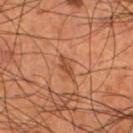image-analysis metrics: an automated nevus-likeness rating near 20 out of 100 and a lesion-detection confidence of about 95/100
patient: male, in their mid-50s
diameter: ≈2.5 mm
site: the left lower leg
image: ~15 mm crop, total-body skin-cancer survey
tile lighting: cross-polarized